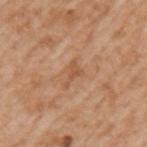biopsy status: imaged on a skin check; not biopsied
lesion diameter: ~3 mm (longest diameter)
image source: ~15 mm crop, total-body skin-cancer survey
automated lesion analysis: a shape eccentricity near 0.85 and two-axis asymmetry of about 0.55; a mean CIELAB color near L≈54 a*≈22 b*≈35, a lesion–skin lightness drop of about 8, and a lesion-to-skin contrast of about 5.5 (normalized; higher = more distinct); an automated nevus-likeness rating near 0 out of 100 and a detector confidence of about 100 out of 100 that the crop contains a lesion
patient: male, roughly 65 years of age
anatomic site: the left upper arm